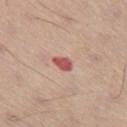Part of a total-body skin-imaging series; this lesion was reviewed on a skin check and was not flagged for biopsy.
A 15 mm crop from a total-body photograph taken for skin-cancer surveillance.
About 2.5 mm across.
The subject is a male aged around 60.
Imaged with white-light lighting.
The lesion is located on the left thigh.
Automated image analysis of the tile measured an area of roughly 3.5 mm² and a symmetry-axis asymmetry near 0.25. The analysis additionally found a mean CIELAB color near L≈55 a*≈30 b*≈25 and about 14 CIELAB-L* units darker than the surrounding skin. The analysis additionally found a within-lesion color-variation index near 1/10 and peripheral color asymmetry of about 0.5. And it measured a detector confidence of about 100 out of 100 that the crop contains a lesion.